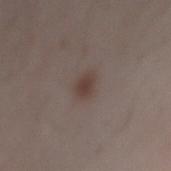Impression: Captured during whole-body skin photography for melanoma surveillance; the lesion was not biopsied.